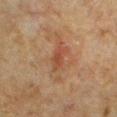{
  "biopsy_status": "not biopsied; imaged during a skin examination",
  "lesion_size": {
    "long_diameter_mm_approx": 3.0
  },
  "site": "chest",
  "image": {
    "source": "total-body photography crop",
    "field_of_view_mm": 15
  },
  "patient": {
    "sex": "male",
    "age_approx": 50
  }
}